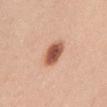patient: female, roughly 45 years of age | tile lighting: white-light illumination | size: about 3.5 mm | body site: the mid back | imaging modality: 15 mm crop, total-body photography.